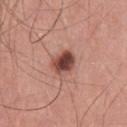workup: catalogued during a skin exam; not biopsied
imaging modality: ~15 mm tile from a whole-body skin photo
site: the front of the torso
patient: male, approximately 60 years of age
lighting: white-light
size: ≈3 mm